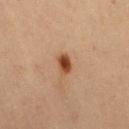Part of a total-body skin-imaging series; this lesion was reviewed on a skin check and was not flagged for biopsy.
The lesion's longest dimension is about 2.5 mm.
On the right thigh.
Automated tile analysis of the lesion measured an average lesion color of about L≈41 a*≈21 b*≈31 (CIELAB). The analysis additionally found internal color variation of about 4 on a 0–10 scale.
This image is a 15 mm lesion crop taken from a total-body photograph.
The subject is a female approximately 70 years of age.